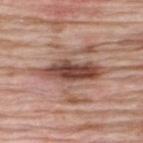{
  "biopsy_status": "not biopsied; imaged during a skin examination",
  "lighting": "white-light",
  "automated_metrics": {
    "area_mm2_approx": 15.0,
    "eccentricity": 0.95,
    "shape_asymmetry": 0.2,
    "border_irregularity_0_10": 4.0,
    "color_variation_0_10": 6.5,
    "peripheral_color_asymmetry": 2.5,
    "nevus_likeness_0_100": 90
  },
  "image": {
    "source": "total-body photography crop",
    "field_of_view_mm": 15
  },
  "site": "back",
  "patient": {
    "sex": "male",
    "age_approx": 60
  }
}